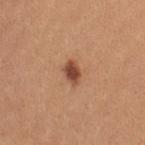Recorded during total-body skin imaging; not selected for excision or biopsy.
Longest diameter approximately 3 mm.
Automated image analysis of the tile measured a mean CIELAB color near L≈48 a*≈25 b*≈32 and a normalized lesion–skin contrast near 10. It also reported border irregularity of about 2 on a 0–10 scale, a color-variation rating of about 2.5/10, and peripheral color asymmetry of about 0.5. And it measured a classifier nevus-likeness of about 95/100 and a lesion-detection confidence of about 100/100.
Captured under white-light illumination.
Cropped from a total-body skin-imaging series; the visible field is about 15 mm.
On the left upper arm.
A female subject, approximately 25 years of age.